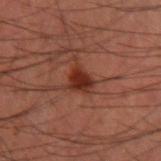Imaged during a routine full-body skin examination; the lesion was not biopsied and no histopathology is available. A 15 mm crop from a total-body photograph taken for skin-cancer surveillance. A male patient, aged 58–62. The lesion's longest dimension is about 3 mm. The lesion is located on the right forearm. The tile uses cross-polarized illumination.